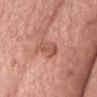follow-up: imaged on a skin check; not biopsied
image: total-body-photography crop, ~15 mm field of view
automated lesion analysis: a shape-asymmetry score of about 0.3 (0 = symmetric); an automated nevus-likeness rating near 0 out of 100 and a detector confidence of about 100 out of 100 that the crop contains a lesion
body site: the front of the torso
patient: female, in their mid-60s